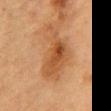Q: How was this image acquired?
A: ~15 mm crop, total-body skin-cancer survey
Q: What are the patient's age and sex?
A: male, in their mid- to late 80s or older
Q: Where on the body is the lesion?
A: the chest
Q: Illumination type?
A: cross-polarized illumination